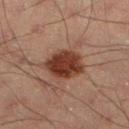Findings:
- biopsy status · no biopsy performed (imaged during a skin exam)
- location · the left lower leg
- automated metrics · a footprint of about 13 mm² and a shape eccentricity near 0.65; an average lesion color of about L≈34 a*≈21 b*≈26 (CIELAB), about 14 CIELAB-L* units darker than the surrounding skin, and a normalized lesion–skin contrast near 12; a border-irregularity rating of about 2/10 and peripheral color asymmetry of about 1
- subject · male, roughly 40 years of age
- image · ~15 mm tile from a whole-body skin photo